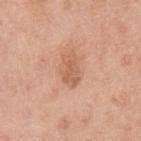Findings:
• workup · total-body-photography surveillance lesion; no biopsy
• lighting · white-light illumination
• lesion diameter · about 3.5 mm
• patient · female, approximately 40 years of age
• image source · ~15 mm crop, total-body skin-cancer survey
• body site · the left upper arm
• automated lesion analysis · a footprint of about 6 mm², an outline eccentricity of about 0.75 (0 = round, 1 = elongated), and a shape-asymmetry score of about 0.2 (0 = symmetric); a lesion color around L≈60 a*≈24 b*≈33 in CIELAB, a lesion–skin lightness drop of about 9, and a normalized border contrast of about 6; a border-irregularity index near 2/10 and a within-lesion color-variation index near 2.5/10